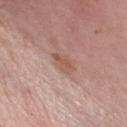Part of a total-body skin-imaging series; this lesion was reviewed on a skin check and was not flagged for biopsy.
A female patient in their 50s.
A roughly 15 mm field-of-view crop from a total-body skin photograph.
From the right lower leg.